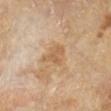The lesion was tiled from a total-body skin photograph and was not biopsied.
Cropped from a total-body skin-imaging series; the visible field is about 15 mm.
About 3 mm across.
From the right lower leg.
Imaged with cross-polarized lighting.
The lesion-visualizer software estimated a lesion color around L≈59 a*≈18 b*≈36 in CIELAB, about 8 CIELAB-L* units darker than the surrounding skin, and a normalized border contrast of about 6. The analysis additionally found a color-variation rating of about 2/10 and peripheral color asymmetry of about 0.5. The software also gave an automated nevus-likeness rating near 0 out of 100 and a detector confidence of about 100 out of 100 that the crop contains a lesion.
The patient is a male roughly 65 years of age.